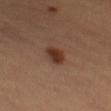Q: Was a biopsy performed?
A: no biopsy performed (imaged during a skin exam)
Q: How large is the lesion?
A: about 3 mm
Q: What is the imaging modality?
A: ~15 mm tile from a whole-body skin photo
Q: What is the anatomic site?
A: the leg
Q: What did automated image analysis measure?
A: an area of roughly 5 mm², an outline eccentricity of about 0.8 (0 = round, 1 = elongated), and a symmetry-axis asymmetry near 0.25; a lesion color around L≈35 a*≈21 b*≈29 in CIELAB, roughly 11 lightness units darker than nearby skin, and a lesion-to-skin contrast of about 10 (normalized; higher = more distinct); internal color variation of about 3 on a 0–10 scale and peripheral color asymmetry of about 1
Q: Illumination type?
A: cross-polarized illumination
Q: Patient demographics?
A: female, aged around 60